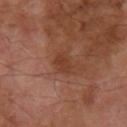Imaged during a routine full-body skin examination; the lesion was not biopsied and no histopathology is available.